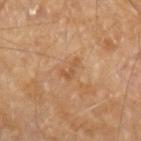The lesion was tiled from a total-body skin photograph and was not biopsied. The lesion is located on the left forearm. Automated tile analysis of the lesion measured a lesion area of about 3 mm², an outline eccentricity of about 0.85 (0 = round, 1 = elongated), and a shape-asymmetry score of about 0.5 (0 = symmetric). It also reported a lesion color around L≈52 a*≈21 b*≈35 in CIELAB and roughly 7 lightness units darker than nearby skin. And it measured internal color variation of about 0 on a 0–10 scale and a peripheral color-asymmetry measure near 0. Captured under cross-polarized illumination. The patient is a male aged 58 to 62. A region of skin cropped from a whole-body photographic capture, roughly 15 mm wide.